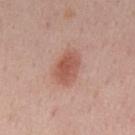Assessment:
Part of a total-body skin-imaging series; this lesion was reviewed on a skin check and was not flagged for biopsy.
Context:
A male subject, aged 53 to 57. The tile uses white-light illumination. A roughly 15 mm field-of-view crop from a total-body skin photograph. On the mid back. Longest diameter approximately 4 mm. An algorithmic analysis of the crop reported border irregularity of about 1.5 on a 0–10 scale and radial color variation of about 1.